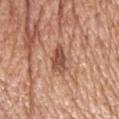The lesion was tiled from a total-body skin photograph and was not biopsied.
From the head or neck.
A region of skin cropped from a whole-body photographic capture, roughly 15 mm wide.
The patient is a male aged around 80.